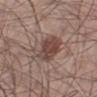| key | value |
|---|---|
| follow-up | total-body-photography surveillance lesion; no biopsy |
| image source | 15 mm crop, total-body photography |
| lighting | white-light illumination |
| body site | the leg |
| subject | male, in their mid- to late 50s |
| TBP lesion metrics | an eccentricity of roughly 0.7; a lesion color around L≈45 a*≈18 b*≈22 in CIELAB, about 11 CIELAB-L* units darker than the surrounding skin, and a normalized border contrast of about 8; a border-irregularity rating of about 3.5/10 and a within-lesion color-variation index near 4/10 |
| diameter | ≈5 mm |